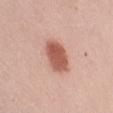The lesion was tiled from a total-body skin photograph and was not biopsied.
The subject is a male about 45 years old.
A 15 mm close-up tile from a total-body photography series done for melanoma screening.
From the right upper arm.